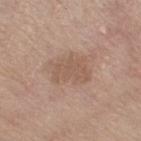A 15 mm close-up tile from a total-body photography series done for melanoma screening. Located on the leg. The patient is a female roughly 65 years of age.